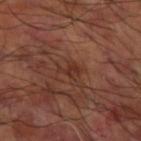<case>
<lesion_size>
  <long_diameter_mm_approx>2.5</long_diameter_mm_approx>
</lesion_size>
<lighting>cross-polarized</lighting>
<patient>
  <sex>male</sex>
  <age_approx>60</age_approx>
</patient>
<image>
  <source>total-body photography crop</source>
  <field_of_view_mm>15</field_of_view_mm>
</image>
<site>right forearm</site>
</case>